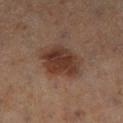acquisition: 15 mm crop, total-body photography
site: the right lower leg
illumination: cross-polarized illumination
lesion size: ~4.5 mm (longest diameter)
patient: female, approximately 55 years of age